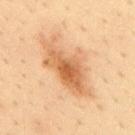The lesion was tiled from a total-body skin photograph and was not biopsied. A roughly 15 mm field-of-view crop from a total-body skin photograph. Automated image analysis of the tile measured an average lesion color of about L≈59 a*≈21 b*≈38 (CIELAB). It also reported a border-irregularity rating of about 5.5/10, a within-lesion color-variation index near 5.5/10, and a peripheral color-asymmetry measure near 1.5. The lesion is on the upper back. A male subject, aged around 35. This is a cross-polarized tile. Longest diameter approximately 8.5 mm.